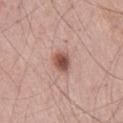follow-up: total-body-photography surveillance lesion; no biopsy | imaging modality: ~15 mm tile from a whole-body skin photo | subject: male, roughly 55 years of age | body site: the right thigh.